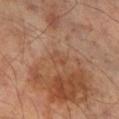Part of a total-body skin-imaging series; this lesion was reviewed on a skin check and was not flagged for biopsy.
The tile uses cross-polarized illumination.
From the right lower leg.
Automated image analysis of the tile measured an outline eccentricity of about 0.8 (0 = round, 1 = elongated) and a shape-asymmetry score of about 0.4 (0 = symmetric). The software also gave a border-irregularity index near 4/10, a color-variation rating of about 0/10, and radial color variation of about 0.
Cropped from a whole-body photographic skin survey; the tile spans about 15 mm.
A male patient in their mid- to late 50s.